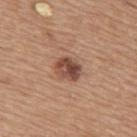No biopsy was performed on this lesion — it was imaged during a full skin examination and was not determined to be concerning. Located on the upper back. The tile uses white-light illumination. A male subject, aged approximately 65. Cropped from a total-body skin-imaging series; the visible field is about 15 mm. The lesion-visualizer software estimated an eccentricity of roughly 0.65 and two-axis asymmetry of about 0.15. The software also gave roughly 14 lightness units darker than nearby skin and a lesion-to-skin contrast of about 10 (normalized; higher = more distinct). And it measured an automated nevus-likeness rating near 70 out of 100 and a lesion-detection confidence of about 100/100.